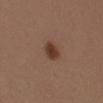The lesion was tiled from a total-body skin photograph and was not biopsied.
A roughly 15 mm field-of-view crop from a total-body skin photograph.
A male patient aged approximately 40.
Longest diameter approximately 3 mm.
The lesion-visualizer software estimated a footprint of about 5 mm², an outline eccentricity of about 0.8 (0 = round, 1 = elongated), and two-axis asymmetry of about 0.15. And it measured roughly 10 lightness units darker than nearby skin and a normalized border contrast of about 9.
This is a white-light tile.
From the mid back.